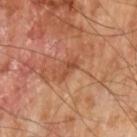Context:
This is a cross-polarized tile. Measured at roughly 3 mm in maximum diameter. A 15 mm crop from a total-body photograph taken for skin-cancer surveillance. On the arm. A male subject about 65 years old.